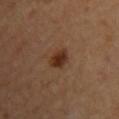Clinical impression: Recorded during total-body skin imaging; not selected for excision or biopsy. Clinical summary: The patient is a male aged 53 to 57. Automated image analysis of the tile measured a lesion area of about 5.5 mm², a shape eccentricity near 0.75, and a symmetry-axis asymmetry near 0.2. The analysis additionally found an average lesion color of about L≈32 a*≈20 b*≈29 (CIELAB) and a normalized border contrast of about 9.5. It also reported border irregularity of about 2 on a 0–10 scale, a color-variation rating of about 5/10, and radial color variation of about 1.5. It also reported a classifier nevus-likeness of about 100/100 and a detector confidence of about 100 out of 100 that the crop contains a lesion. Longest diameter approximately 3 mm. The tile uses cross-polarized illumination. Located on the chest. A lesion tile, about 15 mm wide, cut from a 3D total-body photograph.